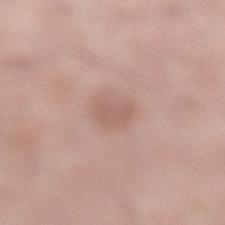Longest diameter approximately 3.5 mm.
The lesion-visualizer software estimated a shape-asymmetry score of about 0.2 (0 = symmetric). The software also gave border irregularity of about 2 on a 0–10 scale, internal color variation of about 2 on a 0–10 scale, and peripheral color asymmetry of about 0.5.
The lesion is on the left lower leg.
A region of skin cropped from a whole-body photographic capture, roughly 15 mm wide.
A male subject, aged approximately 60.